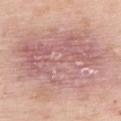biopsy status = imaged on a skin check; not biopsied
subject = female, aged around 50
diameter = ~13 mm (longest diameter)
image = ~15 mm crop, total-body skin-cancer survey
automated lesion analysis = an average lesion color of about L≈63 a*≈22 b*≈22 (CIELAB), roughly 10 lightness units darker than nearby skin, and a normalized border contrast of about 7
location = the upper back